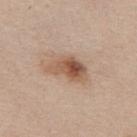<record>
<biopsy_status>not biopsied; imaged during a skin examination</biopsy_status>
<lighting>white-light</lighting>
<image>
  <source>total-body photography crop</source>
  <field_of_view_mm>15</field_of_view_mm>
</image>
<automated_metrics>
  <eccentricity>0.85</eccentricity>
  <shape_asymmetry>0.3</shape_asymmetry>
  <cielab_L>55</cielab_L>
  <cielab_a>19</cielab_a>
  <cielab_b>30</cielab_b>
  <vs_skin_darker_L>12.0</vs_skin_darker_L>
  <vs_skin_contrast_norm>8.5</vs_skin_contrast_norm>
  <color_variation_0_10>9.0</color_variation_0_10>
  <peripheral_color_asymmetry>3.5</peripheral_color_asymmetry>
  <nevus_likeness_0_100>85</nevus_likeness_0_100>
  <lesion_detection_confidence_0_100>100</lesion_detection_confidence_0_100>
</automated_metrics>
<patient>
  <sex>female</sex>
  <age_approx>55</age_approx>
</patient>
<lesion_size>
  <long_diameter_mm_approx>5.0</long_diameter_mm_approx>
</lesion_size>
<site>upper back</site>
</record>